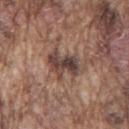Imaged during a routine full-body skin examination; the lesion was not biopsied and no histopathology is available.